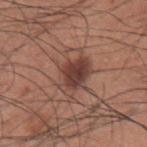Findings:
– biopsy status: imaged on a skin check; not biopsied
– imaging modality: ~15 mm tile from a whole-body skin photo
– tile lighting: white-light
– location: the left upper arm
– diameter: ≈6 mm
– subject: male, about 35 years old